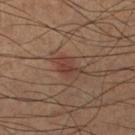{"biopsy_status": "not biopsied; imaged during a skin examination", "lighting": "cross-polarized", "lesion_size": {"long_diameter_mm_approx": 4.0}, "patient": {"sex": "male", "age_approx": 60}, "site": "right lower leg", "automated_metrics": {"border_irregularity_0_10": 3.5, "peripheral_color_asymmetry": 1.0}, "image": {"source": "total-body photography crop", "field_of_view_mm": 15}}